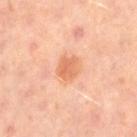Assessment:
This lesion was catalogued during total-body skin photography and was not selected for biopsy.
Acquisition and patient details:
A female patient roughly 50 years of age. This is a cross-polarized tile. An algorithmic analysis of the crop reported a lesion color around L≈66 a*≈26 b*≈37 in CIELAB and about 9 CIELAB-L* units darker than the surrounding skin. And it measured internal color variation of about 3 on a 0–10 scale and peripheral color asymmetry of about 1. A 15 mm crop from a total-body photograph taken for skin-cancer surveillance. The lesion is located on the right thigh.